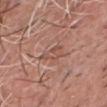biopsy status: catalogued during a skin exam; not biopsied
tile lighting: white-light
lesion diameter: ≈3 mm
subject: male, aged 48–52
imaging modality: total-body-photography crop, ~15 mm field of view
anatomic site: the front of the torso